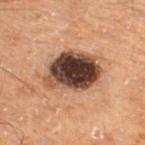Assessment:
No biopsy was performed on this lesion — it was imaged during a full skin examination and was not determined to be concerning.
Acquisition and patient details:
A male subject, approximately 70 years of age. Captured under cross-polarized illumination. Automated image analysis of the tile measured internal color variation of about 8.5 on a 0–10 scale and a peripheral color-asymmetry measure near 2.5. The recorded lesion diameter is about 5.5 mm. A region of skin cropped from a whole-body photographic capture, roughly 15 mm wide. Located on the left thigh.